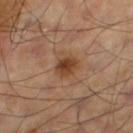Impression:
Captured during whole-body skin photography for melanoma surveillance; the lesion was not biopsied.